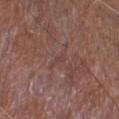No biopsy was performed on this lesion — it was imaged during a full skin examination and was not determined to be concerning. A male subject approximately 75 years of age. The recorded lesion diameter is about 1 mm. The lesion is on the right lower leg. Imaged with white-light lighting. Cropped from a total-body skin-imaging series; the visible field is about 15 mm.